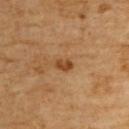Q: Was this lesion biopsied?
A: total-body-photography surveillance lesion; no biopsy
Q: Lesion location?
A: the upper back
Q: What lighting was used for the tile?
A: cross-polarized illumination
Q: What are the patient's age and sex?
A: male, approximately 60 years of age
Q: What is the lesion's diameter?
A: ≈2.5 mm
Q: What kind of image is this?
A: 15 mm crop, total-body photography